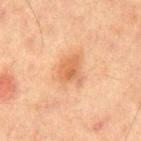Context:
The tile uses cross-polarized illumination. Longest diameter approximately 3.5 mm. The lesion is on the chest. The patient is a male aged 63 to 67. A 15 mm crop from a total-body photograph taken for skin-cancer surveillance.